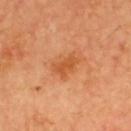| field | value |
|---|---|
| follow-up | no biopsy performed (imaged during a skin exam) |
| anatomic site | the upper back |
| lesion diameter | about 3.5 mm |
| patient | male, approximately 65 years of age |
| imaging modality | ~15 mm tile from a whole-body skin photo |